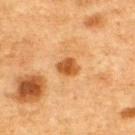notes = total-body-photography surveillance lesion; no biopsy
anatomic site = the upper back
subject = male, approximately 75 years of age
image = 15 mm crop, total-body photography